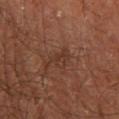Captured during whole-body skin photography for melanoma surveillance; the lesion was not biopsied.
On the left lower leg.
A 15 mm close-up tile from a total-body photography series done for melanoma screening.
Automated tile analysis of the lesion measured an area of roughly 5 mm² and an eccentricity of roughly 0.9. And it measured peripheral color asymmetry of about 1.
A male patient aged 63–67.
Approximately 4 mm at its widest.
Imaged with cross-polarized lighting.